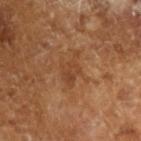Assessment: Part of a total-body skin-imaging series; this lesion was reviewed on a skin check and was not flagged for biopsy. Acquisition and patient details: A lesion tile, about 15 mm wide, cut from a 3D total-body photograph. The lesion's longest dimension is about 4 mm. The tile uses cross-polarized illumination. A male patient aged 63–67. The total-body-photography lesion software estimated border irregularity of about 5.5 on a 0–10 scale, internal color variation of about 2.5 on a 0–10 scale, and a peripheral color-asymmetry measure near 0.5. The software also gave a nevus-likeness score of about 0/100 and a lesion-detection confidence of about 100/100.